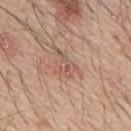Clinical impression: Part of a total-body skin-imaging series; this lesion was reviewed on a skin check and was not flagged for biopsy. Image and clinical context: Captured under white-light illumination. A lesion tile, about 15 mm wide, cut from a 3D total-body photograph. A male patient, aged around 65. Automated image analysis of the tile measured a footprint of about 5.5 mm² and two-axis asymmetry of about 0.5. It also reported a lesion color around L≈56 a*≈20 b*≈27 in CIELAB, about 7 CIELAB-L* units darker than the surrounding skin, and a lesion-to-skin contrast of about 5 (normalized; higher = more distinct). From the upper back. Measured at roughly 3.5 mm in maximum diameter.